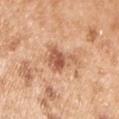The lesion was photographed on a routine skin check and not biopsied; there is no pathology result. The patient is a male approximately 55 years of age. A 15 mm close-up extracted from a 3D total-body photography capture. Imaged with white-light lighting. Located on the left upper arm.